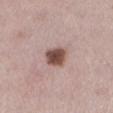Q: Is there a histopathology result?
A: total-body-photography surveillance lesion; no biopsy
Q: What did automated image analysis measure?
A: a normalized lesion–skin contrast near 11; an automated nevus-likeness rating near 90 out of 100 and a lesion-detection confidence of about 100/100
Q: Illumination type?
A: white-light
Q: Patient demographics?
A: female, aged 43 to 47
Q: Where on the body is the lesion?
A: the left lower leg
Q: How large is the lesion?
A: about 3 mm
Q: What kind of image is this?
A: 15 mm crop, total-body photography